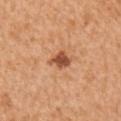{"biopsy_status": "not biopsied; imaged during a skin examination", "patient": {"sex": "male", "age_approx": 55}, "site": "right upper arm", "lighting": "white-light", "image": {"source": "total-body photography crop", "field_of_view_mm": 15}}